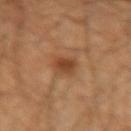This lesion was catalogued during total-body skin photography and was not selected for biopsy.
About 2.5 mm across.
A close-up tile cropped from a whole-body skin photograph, about 15 mm across.
A male patient roughly 45 years of age.
This is a cross-polarized tile.
From the left forearm.